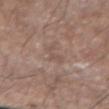Impression:
This lesion was catalogued during total-body skin photography and was not selected for biopsy.
Image and clinical context:
The patient is a male roughly 75 years of age. On the left forearm. The recorded lesion diameter is about 3 mm. The total-body-photography lesion software estimated a footprint of about 3.5 mm², an outline eccentricity of about 0.9 (0 = round, 1 = elongated), and a shape-asymmetry score of about 0.5 (0 = symmetric). The software also gave about 6 CIELAB-L* units darker than the surrounding skin and a normalized lesion–skin contrast near 4.5. The analysis additionally found a border-irregularity rating of about 6.5/10, a within-lesion color-variation index near 0.5/10, and peripheral color asymmetry of about 0. It also reported a classifier nevus-likeness of about 0/100. A 15 mm close-up tile from a total-body photography series done for melanoma screening.